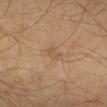<lesion>
<biopsy_status>not biopsied; imaged during a skin examination</biopsy_status>
<patient>
  <sex>male</sex>
  <age_approx>40</age_approx>
</patient>
<site>right lower leg</site>
<lesion_size>
  <long_diameter_mm_approx>2.5</long_diameter_mm_approx>
</lesion_size>
<lighting>cross-polarized</lighting>
<automated_metrics>
  <cielab_L>54</cielab_L>
  <cielab_a>17</cielab_a>
  <cielab_b>34</cielab_b>
  <vs_skin_contrast_norm>4.5</vs_skin_contrast_norm>
  <border_irregularity_0_10>4.0</border_irregularity_0_10>
  <color_variation_0_10>0.5</color_variation_0_10>
  <peripheral_color_asymmetry>0.0</peripheral_color_asymmetry>
</automated_metrics>
<image>
  <source>total-body photography crop</source>
  <field_of_view_mm>15</field_of_view_mm>
</image>
</lesion>